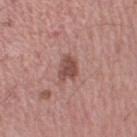biopsy status: catalogued during a skin exam; not biopsied | subject: male, aged 43 to 47 | automated metrics: a footprint of about 5 mm², an outline eccentricity of about 0.7 (0 = round, 1 = elongated), and a symmetry-axis asymmetry near 0.3; an average lesion color of about L≈48 a*≈23 b*≈23 (CIELAB) and a lesion–skin lightness drop of about 12; an automated nevus-likeness rating near 45 out of 100 and a detector confidence of about 100 out of 100 that the crop contains a lesion | site: the left upper arm | image: 15 mm crop, total-body photography | size: about 3 mm.